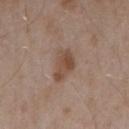| field | value |
|---|---|
| acquisition | ~15 mm tile from a whole-body skin photo |
| illumination | white-light illumination |
| subject | male, aged 53 to 57 |
| site | the right upper arm |